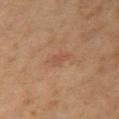Case summary:
- biopsy status: no biopsy performed (imaged during a skin exam)
- patient: female, in their mid- to late 60s
- size: ~2.5 mm (longest diameter)
- image source: ~15 mm tile from a whole-body skin photo
- TBP lesion metrics: a lesion area of about 3 mm², an eccentricity of roughly 0.85, and a shape-asymmetry score of about 0.3 (0 = symmetric); a mean CIELAB color near L≈50 a*≈21 b*≈30, a lesion–skin lightness drop of about 6, and a normalized lesion–skin contrast near 4.5; lesion-presence confidence of about 100/100
- anatomic site: the arm
- lighting: cross-polarized